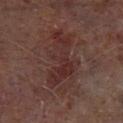• biopsy status: imaged on a skin check; not biopsied